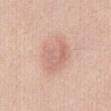{
  "biopsy_status": "not biopsied; imaged during a skin examination",
  "image": {
    "source": "total-body photography crop",
    "field_of_view_mm": 15
  },
  "site": "abdomen",
  "patient": {
    "sex": "female",
    "age_approx": 45
  }
}